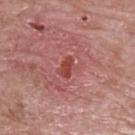Captured during whole-body skin photography for melanoma surveillance; the lesion was not biopsied. A male patient, roughly 60 years of age. On the left upper arm. A 15 mm crop from a total-body photograph taken for skin-cancer surveillance.